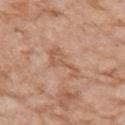Automated image analysis of the tile measured an area of roughly 6 mm², a shape eccentricity near 0.95, and a symmetry-axis asymmetry near 0.6. The analysis additionally found a lesion color around L≈57 a*≈21 b*≈33 in CIELAB, about 7 CIELAB-L* units darker than the surrounding skin, and a lesion-to-skin contrast of about 5.5 (normalized; higher = more distinct). The software also gave a border-irregularity rating of about 8/10, internal color variation of about 2 on a 0–10 scale, and a peripheral color-asymmetry measure near 0.5. Longest diameter approximately 5 mm. A female subject in their mid-70s. The lesion is on the right upper arm. A roughly 15 mm field-of-view crop from a total-body skin photograph.